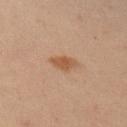<case>
<biopsy_status>not biopsied; imaged during a skin examination</biopsy_status>
<site>right upper arm</site>
<image>
  <source>total-body photography crop</source>
  <field_of_view_mm>15</field_of_view_mm>
</image>
<lesion_size>
  <long_diameter_mm_approx>3.0</long_diameter_mm_approx>
</lesion_size>
<automated_metrics>
  <area_mm2_approx>4.0</area_mm2_approx>
  <eccentricity>0.85</eccentricity>
  <shape_asymmetry>0.3</shape_asymmetry>
  <border_irregularity_0_10>3.0</border_irregularity_0_10>
  <color_variation_0_10>2.0</color_variation_0_10>
  <peripheral_color_asymmetry>1.0</peripheral_color_asymmetry>
  <nevus_likeness_0_100>80</nevus_likeness_0_100>
  <lesion_detection_confidence_0_100>100</lesion_detection_confidence_0_100>
</automated_metrics>
<patient>
  <sex>male</sex>
  <age_approx>50</age_approx>
</patient>
<lighting>cross-polarized</lighting>
</case>